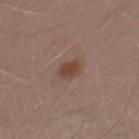Recorded during total-body skin imaging; not selected for excision or biopsy.
About 3 mm across.
A male subject, approximately 30 years of age.
Automated image analysis of the tile measured a lesion color around L≈43 a*≈18 b*≈25 in CIELAB and roughly 9 lightness units darker than nearby skin.
A close-up tile cropped from a whole-body skin photograph, about 15 mm across.
The lesion is on the left thigh.